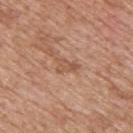Q: Was this lesion biopsied?
A: total-body-photography surveillance lesion; no biopsy
Q: Patient demographics?
A: male, approximately 60 years of age
Q: Lesion size?
A: ≈2.5 mm
Q: What did automated image analysis measure?
A: a footprint of about 3 mm² and a symmetry-axis asymmetry near 0.5; border irregularity of about 5 on a 0–10 scale, internal color variation of about 1 on a 0–10 scale, and radial color variation of about 0.5
Q: What lighting was used for the tile?
A: white-light
Q: What is the imaging modality?
A: ~15 mm crop, total-body skin-cancer survey
Q: Lesion location?
A: the upper back